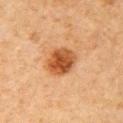biopsy status=catalogued during a skin exam; not biopsied